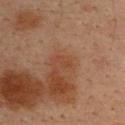No biopsy was performed on this lesion — it was imaged during a full skin examination and was not determined to be concerning. An algorithmic analysis of the crop reported an outline eccentricity of about 0.85 (0 = round, 1 = elongated) and a shape-asymmetry score of about 0.45 (0 = symmetric). And it measured about 5 CIELAB-L* units darker than the surrounding skin and a normalized lesion–skin contrast near 5. It also reported border irregularity of about 5 on a 0–10 scale and internal color variation of about 0 on a 0–10 scale. The tile uses cross-polarized illumination. A region of skin cropped from a whole-body photographic capture, roughly 15 mm wide. About 2.5 mm across. From the upper back. A male subject, aged approximately 35.